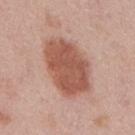biopsy_status: not biopsied; imaged during a skin examination
patient:
  sex: male
  age_approx: 45
lighting: white-light
site: mid back
image:
  source: total-body photography crop
  field_of_view_mm: 15